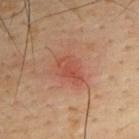Biopsy histopathology demonstrated a nodular basal cell carcinoma.